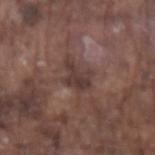biopsy_status: not biopsied; imaged during a skin examination
site: left forearm
patient:
  sex: male
  age_approx: 75
image:
  source: total-body photography crop
  field_of_view_mm: 15
lighting: white-light
lesion_size:
  long_diameter_mm_approx: 3.5